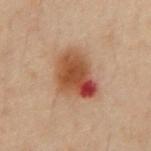| feature | finding |
|---|---|
| follow-up | no biopsy performed (imaged during a skin exam) |
| patient | male, aged around 50 |
| size | about 5 mm |
| body site | the chest |
| lighting | cross-polarized |
| automated lesion analysis | a lesion area of about 17 mm²; an average lesion color of about L≈37 a*≈19 b*≈26 (CIELAB) and a normalized lesion–skin contrast near 10; a border-irregularity index near 3/10, internal color variation of about 10 on a 0–10 scale, and radial color variation of about 3.5; a nevus-likeness score of about 0/100 |
| image source | total-body-photography crop, ~15 mm field of view |